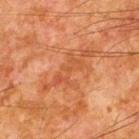Notes:
– follow-up: imaged on a skin check; not biopsied
– acquisition: 15 mm crop, total-body photography
– subject: male, aged approximately 65
– location: the back